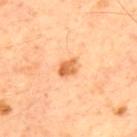This lesion was catalogued during total-body skin photography and was not selected for biopsy. This image is a 15 mm lesion crop taken from a total-body photograph. Located on the upper back. A male subject roughly 60 years of age. The recorded lesion diameter is about 2.5 mm. The tile uses cross-polarized illumination.